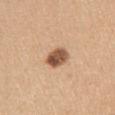Impression: The lesion was tiled from a total-body skin photograph and was not biopsied. Image and clinical context: Located on the left upper arm. Captured under white-light illumination. The recorded lesion diameter is about 3.5 mm. Automated tile analysis of the lesion measured a lesion area of about 6.5 mm², an outline eccentricity of about 0.65 (0 = round, 1 = elongated), and a shape-asymmetry score of about 0.15 (0 = symmetric). And it measured a color-variation rating of about 5.5/10 and a peripheral color-asymmetry measure near 2. A female subject roughly 30 years of age. A lesion tile, about 15 mm wide, cut from a 3D total-body photograph.